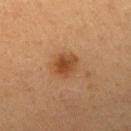The lesion was photographed on a routine skin check and not biopsied; there is no pathology result. A female subject aged approximately 40. Located on the right upper arm. The lesion-visualizer software estimated a classifier nevus-likeness of about 95/100. A roughly 15 mm field-of-view crop from a total-body skin photograph.